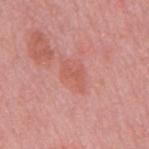follow-up: imaged on a skin check; not biopsied
TBP lesion metrics: an outline eccentricity of about 0.8 (0 = round, 1 = elongated); a mean CIELAB color near L≈58 a*≈29 b*≈28, about 7 CIELAB-L* units darker than the surrounding skin, and a normalized lesion–skin contrast near 5; a border-irregularity index near 5.5/10, internal color variation of about 2 on a 0–10 scale, and peripheral color asymmetry of about 0.5; an automated nevus-likeness rating near 0 out of 100 and a detector confidence of about 100 out of 100 that the crop contains a lesion
diameter: ≈3.5 mm
tile lighting: white-light illumination
subject: male, approximately 50 years of age
imaging modality: total-body-photography crop, ~15 mm field of view
site: the back